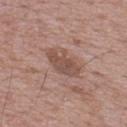<tbp_lesion>
  <biopsy_status>not biopsied; imaged during a skin examination</biopsy_status>
  <lighting>white-light</lighting>
  <lesion_size>
    <long_diameter_mm_approx>4.5</long_diameter_mm_approx>
  </lesion_size>
  <patient>
    <sex>male</sex>
    <age_approx>70</age_approx>
  </patient>
  <site>upper back</site>
  <image>
    <source>total-body photography crop</source>
    <field_of_view_mm>15</field_of_view_mm>
  </image>
</tbp_lesion>